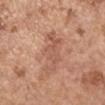The lesion was photographed on a routine skin check and not biopsied; there is no pathology result. A female subject, about 65 years old. The recorded lesion diameter is about 6 mm. From the left upper arm. Imaged with white-light lighting. Cropped from a total-body skin-imaging series; the visible field is about 15 mm.